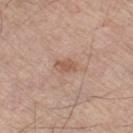Case summary:
– follow-up · imaged on a skin check; not biopsied
– acquisition · ~15 mm crop, total-body skin-cancer survey
– site · the left thigh
– automated lesion analysis · a lesion area of about 4 mm² and two-axis asymmetry of about 0.2; an average lesion color of about L≈56 a*≈20 b*≈29 (CIELAB), roughly 8 lightness units darker than nearby skin, and a normalized lesion–skin contrast near 6; border irregularity of about 2 on a 0–10 scale, internal color variation of about 1.5 on a 0–10 scale, and a peripheral color-asymmetry measure near 0.5
– lesion diameter · ~2.5 mm (longest diameter)
– illumination · white-light
– patient · male, aged 63–67